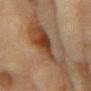Located on the left forearm. The subject is a female aged around 80. A 15 mm close-up extracted from a 3D total-body photography capture.